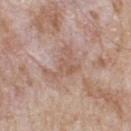- biopsy status: imaged on a skin check; not biopsied
- lesion diameter: ≈4.5 mm
- image: ~15 mm crop, total-body skin-cancer survey
- tile lighting: white-light
- site: the upper back
- TBP lesion metrics: border irregularity of about 10 on a 0–10 scale and a color-variation rating of about 2/10; an automated nevus-likeness rating near 0 out of 100 and a lesion-detection confidence of about 100/100
- subject: male, aged 78 to 82